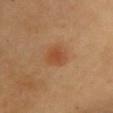Assessment:
Recorded during total-body skin imaging; not selected for excision or biopsy.
Acquisition and patient details:
Approximately 3 mm at its widest. The total-body-photography lesion software estimated a border-irregularity rating of about 2/10 and radial color variation of about 0.5. The software also gave an automated nevus-likeness rating near 85 out of 100. The patient is a female in their mid-60s. A close-up tile cropped from a whole-body skin photograph, about 15 mm across. This is a cross-polarized tile. The lesion is on the chest.